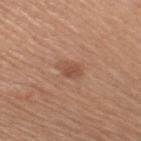No biopsy was performed on this lesion — it was imaged during a full skin examination and was not determined to be concerning. The subject is a female aged 63–67. Measured at roughly 2.5 mm in maximum diameter. Automated tile analysis of the lesion measured an average lesion color of about L≈50 a*≈22 b*≈30 (CIELAB), roughly 9 lightness units darker than nearby skin, and a lesion-to-skin contrast of about 6 (normalized; higher = more distinct). The analysis additionally found a nevus-likeness score of about 5/100 and a detector confidence of about 100 out of 100 that the crop contains a lesion. Captured under white-light illumination. The lesion is on the left arm. A close-up tile cropped from a whole-body skin photograph, about 15 mm across.